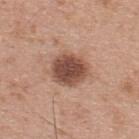<lesion>
<biopsy_status>not biopsied; imaged during a skin examination</biopsy_status>
<site>upper back</site>
<image>
  <source>total-body photography crop</source>
  <field_of_view_mm>15</field_of_view_mm>
</image>
<patient>
  <sex>male</sex>
  <age_approx>30</age_approx>
</patient>
</lesion>